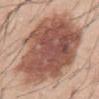{"biopsy_status": "not biopsied; imaged during a skin examination", "automated_metrics": {"eccentricity": 0.55, "cielab_L": 53, "cielab_a": 22, "cielab_b": 27, "vs_skin_darker_L": 17.0, "vs_skin_contrast_norm": 11.0, "nevus_likeness_0_100": 95, "lesion_detection_confidence_0_100": 100}, "lighting": "white-light", "patient": {"sex": "male", "age_approx": 45}, "image": {"source": "total-body photography crop", "field_of_view_mm": 15}, "site": "abdomen"}